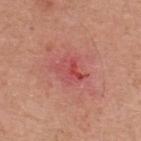| field | value |
|---|---|
| biopsy status | no biopsy performed (imaged during a skin exam) |
| image source | ~15 mm tile from a whole-body skin photo |
| illumination | white-light |
| body site | the back |
| subject | male, aged approximately 55 |